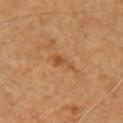Q: What is the anatomic site?
A: the front of the torso
Q: What lighting was used for the tile?
A: cross-polarized illumination
Q: What are the patient's age and sex?
A: male, aged 63 to 67
Q: How was this image acquired?
A: total-body-photography crop, ~15 mm field of view